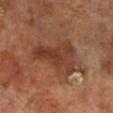{
  "biopsy_status": "not biopsied; imaged during a skin examination"
}